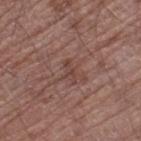  biopsy_status: not biopsied; imaged during a skin examination
  lighting: white-light
  image:
    source: total-body photography crop
    field_of_view_mm: 15
  lesion_size:
    long_diameter_mm_approx: 3.0
  site: left thigh
  patient:
    sex: male
    age_approx: 70